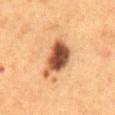notes — catalogued during a skin exam; not biopsied | body site — the abdomen | automated metrics — a lesion color around L≈45 a*≈20 b*≈31 in CIELAB, a lesion–skin lightness drop of about 17, and a lesion-to-skin contrast of about 12 (normalized; higher = more distinct) | acquisition — ~15 mm crop, total-body skin-cancer survey | diameter — ≈5.5 mm | subject — female, aged approximately 50.